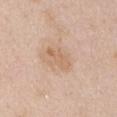Clinical impression:
The lesion was tiled from a total-body skin photograph and was not biopsied.
Background:
Imaged with white-light lighting. This image is a 15 mm lesion crop taken from a total-body photograph. About 3.5 mm across. The total-body-photography lesion software estimated a lesion area of about 4.5 mm² and a shape eccentricity near 0.9. It also reported a lesion color around L≈64 a*≈18 b*≈33 in CIELAB, roughly 7 lightness units darker than nearby skin, and a lesion-to-skin contrast of about 5.5 (normalized; higher = more distinct). A female subject aged approximately 65. Located on the abdomen.